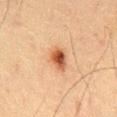{
  "patient": {
    "sex": "male",
    "age_approx": 60
  },
  "image": {
    "source": "total-body photography crop",
    "field_of_view_mm": 15
  },
  "automated_metrics": {
    "area_mm2_approx": 5.5,
    "eccentricity": 0.7,
    "shape_asymmetry": 0.25,
    "cielab_L": 44,
    "cielab_a": 21,
    "cielab_b": 31,
    "vs_skin_darker_L": 13.0
  },
  "site": "abdomen",
  "lesion_size": {
    "long_diameter_mm_approx": 3.0
  },
  "lighting": "cross-polarized"
}